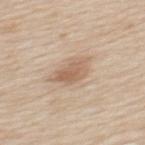Findings:
• notes · imaged on a skin check; not biopsied
• automated metrics · a lesion area of about 7 mm², a shape eccentricity near 0.8, and a symmetry-axis asymmetry near 0.35; a mean CIELAB color near L≈61 a*≈17 b*≈30 and a lesion–skin lightness drop of about 10; a border-irregularity index near 3.5/10, a color-variation rating of about 3.5/10, and peripheral color asymmetry of about 1.5; a nevus-likeness score of about 70/100 and lesion-presence confidence of about 100/100
• subject · male, about 60 years old
• body site · the mid back
• illumination · white-light
• size · about 4 mm
• image source · 15 mm crop, total-body photography